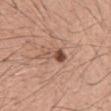Captured during whole-body skin photography for melanoma surveillance; the lesion was not biopsied.
Cropped from a total-body skin-imaging series; the visible field is about 15 mm.
Longest diameter approximately 3.5 mm.
A male subject in their mid- to late 40s.
Captured under white-light illumination.
The lesion is on the mid back.
Automated tile analysis of the lesion measured a border-irregularity index near 3.5/10, a within-lesion color-variation index near 3.5/10, and peripheral color asymmetry of about 1. It also reported a nevus-likeness score of about 80/100 and a detector confidence of about 100 out of 100 that the crop contains a lesion.